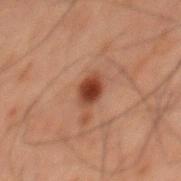* notes — no biopsy performed (imaged during a skin exam)
* location — the mid back
* subject — male, aged 58–62
* automated lesion analysis — border irregularity of about 1.5 on a 0–10 scale
* imaging modality — 15 mm crop, total-body photography
* illumination — cross-polarized
* lesion diameter — about 3 mm